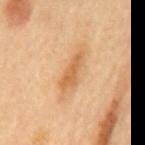<lesion>
<biopsy_status>not biopsied; imaged during a skin examination</biopsy_status>
<image>
  <source>total-body photography crop</source>
  <field_of_view_mm>15</field_of_view_mm>
</image>
<lighting>cross-polarized</lighting>
<patient>
  <sex>male</sex>
  <age_approx>85+</age_approx>
</patient>
<lesion_size>
  <long_diameter_mm_approx>4.5</long_diameter_mm_approx>
</lesion_size>
<site>mid back</site>
</lesion>